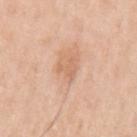Impression:
This lesion was catalogued during total-body skin photography and was not selected for biopsy.
Context:
The lesion is on the left upper arm. Measured at roughly 2.5 mm in maximum diameter. The tile uses white-light illumination. A male patient roughly 65 years of age. This image is a 15 mm lesion crop taken from a total-body photograph.